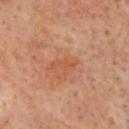No biopsy was performed on this lesion — it was imaged during a full skin examination and was not determined to be concerning. Longest diameter approximately 3.5 mm. A close-up tile cropped from a whole-body skin photograph, about 15 mm across. A male patient, aged 63–67. Captured under cross-polarized illumination. On the head or neck.